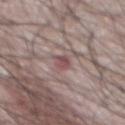No biopsy was performed on this lesion — it was imaged during a full skin examination and was not determined to be concerning.